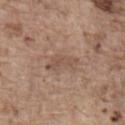Q: Was this lesion biopsied?
A: catalogued during a skin exam; not biopsied
Q: Automated lesion metrics?
A: a mean CIELAB color near L≈51 a*≈18 b*≈26, a lesion–skin lightness drop of about 7, and a lesion-to-skin contrast of about 5 (normalized; higher = more distinct); a border-irregularity rating of about 5/10; a classifier nevus-likeness of about 0/100 and lesion-presence confidence of about 100/100
Q: How was this image acquired?
A: ~15 mm tile from a whole-body skin photo
Q: Illumination type?
A: white-light illumination
Q: What are the patient's age and sex?
A: male, roughly 80 years of age
Q: How large is the lesion?
A: ≈4 mm
Q: What is the anatomic site?
A: the back